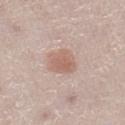Clinical impression:
Part of a total-body skin-imaging series; this lesion was reviewed on a skin check and was not flagged for biopsy.
Background:
The lesion is located on the left lower leg. The recorded lesion diameter is about 3.5 mm. A roughly 15 mm field-of-view crop from a total-body skin photograph. A female patient, approximately 50 years of age.